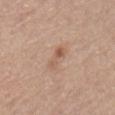Automated tile analysis of the lesion measured a lesion area of about 3.5 mm², an outline eccentricity of about 0.9 (0 = round, 1 = elongated), and a shape-asymmetry score of about 0.4 (0 = symmetric). And it measured a lesion color around L≈57 a*≈19 b*≈30 in CIELAB, about 8 CIELAB-L* units darker than the surrounding skin, and a lesion-to-skin contrast of about 6 (normalized; higher = more distinct). The software also gave border irregularity of about 5 on a 0–10 scale, a color-variation rating of about 0.5/10, and peripheral color asymmetry of about 0. The software also gave an automated nevus-likeness rating near 10 out of 100 and a lesion-detection confidence of about 100/100.
Captured under white-light illumination.
The recorded lesion diameter is about 3 mm.
A close-up tile cropped from a whole-body skin photograph, about 15 mm across.
A male subject aged around 80.
On the mid back.